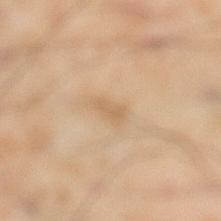Assessment: The lesion was tiled from a total-body skin photograph and was not biopsied. Image and clinical context: Approximately 3 mm at its widest. A 15 mm crop from a total-body photograph taken for skin-cancer surveillance. Automated image analysis of the tile measured two-axis asymmetry of about 0.35. The subject is a male roughly 55 years of age. On the left lower leg.